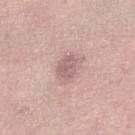workup = imaged on a skin check; not biopsied
subject = female, approximately 50 years of age
automated lesion analysis = a footprint of about 5 mm² and a symmetry-axis asymmetry near 0.2; border irregularity of about 2 on a 0–10 scale, a within-lesion color-variation index near 2/10, and radial color variation of about 0.5; a classifier nevus-likeness of about 0/100 and a lesion-detection confidence of about 100/100
location = the leg
diameter = ≈3 mm
image = ~15 mm tile from a whole-body skin photo
illumination = white-light